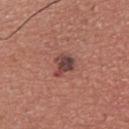biopsy_status: not biopsied; imaged during a skin examination
image:
  source: total-body photography crop
  field_of_view_mm: 15
lighting: white-light
lesion_size:
  long_diameter_mm_approx: 3.0
automated_metrics:
  area_mm2_approx: 5.5
  shape_asymmetry: 0.45
  border_irregularity_0_10: 4.5
  color_variation_0_10: 4.0
  peripheral_color_asymmetry: 1.5
patient:
  sex: male
  age_approx: 40
site: upper back